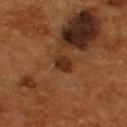site=the upper back
lesion size=~2.5 mm (longest diameter)
acquisition=~15 mm crop, total-body skin-cancer survey
subject=male, aged 53–57
tile lighting=cross-polarized illumination